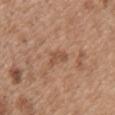image source: total-body-photography crop, ~15 mm field of view; lesion diameter: ~3 mm (longest diameter); patient: male, aged around 50; location: the back.